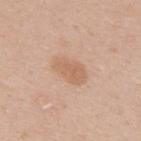Findings:
- notes · total-body-photography surveillance lesion; no biopsy
- image · total-body-photography crop, ~15 mm field of view
- tile lighting · white-light illumination
- patient · female, aged 28–32
- location · the back
- diameter · about 4 mm